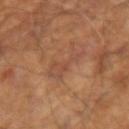Q: Was this lesion biopsied?
A: total-body-photography surveillance lesion; no biopsy
Q: Automated lesion metrics?
A: a nevus-likeness score of about 0/100 and lesion-presence confidence of about 95/100
Q: Lesion location?
A: the right upper arm
Q: How large is the lesion?
A: ≈4 mm
Q: Who is the patient?
A: male, aged 63–67
Q: Illumination type?
A: cross-polarized
Q: What is the imaging modality?
A: ~15 mm crop, total-body skin-cancer survey